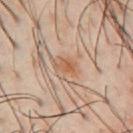Recorded during total-body skin imaging; not selected for excision or biopsy.
Imaged with cross-polarized lighting.
The lesion-visualizer software estimated a footprint of about 4 mm², an eccentricity of roughly 0.75, and two-axis asymmetry of about 0.35. And it measured a classifier nevus-likeness of about 60/100.
A close-up tile cropped from a whole-body skin photograph, about 15 mm across.
The lesion's longest dimension is about 2.5 mm.
A male subject, aged 38–42.
On the chest.